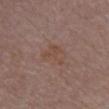The lesion was tiled from a total-body skin photograph and was not biopsied.
Located on the chest.
Cropped from a whole-body photographic skin survey; the tile spans about 15 mm.
The subject is a female about 80 years old.
An algorithmic analysis of the crop reported a footprint of about 6 mm² and an outline eccentricity of about 0.55 (0 = round, 1 = elongated). The analysis additionally found a lesion color around L≈46 a*≈18 b*≈25 in CIELAB, a lesion–skin lightness drop of about 5, and a lesion-to-skin contrast of about 5 (normalized; higher = more distinct). It also reported a border-irregularity index near 4.5/10 and a within-lesion color-variation index near 2.5/10.
The lesion's longest dimension is about 3 mm.
This is a white-light tile.